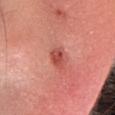notes — total-body-photography surveillance lesion; no biopsy | body site — the head or neck | image source — ~15 mm crop, total-body skin-cancer survey | patient — female, approximately 35 years of age.